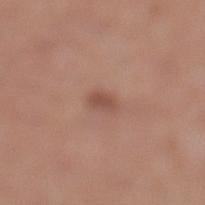| feature | finding |
|---|---|
| workup | total-body-photography surveillance lesion; no biopsy |
| TBP lesion metrics | an area of roughly 3 mm² |
| site | the left lower leg |
| illumination | white-light illumination |
| subject | female, aged 28–32 |
| acquisition | ~15 mm tile from a whole-body skin photo |
| lesion size | ≈2.5 mm |